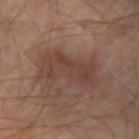Q: Was this lesion biopsied?
A: no biopsy performed (imaged during a skin exam)
Q: Patient demographics?
A: male, aged 68 to 72
Q: What is the imaging modality?
A: 15 mm crop, total-body photography
Q: What did automated image analysis measure?
A: a lesion area of about 16 mm², a shape eccentricity near 0.85, and a shape-asymmetry score of about 0.35 (0 = symmetric); a mean CIELAB color near L≈39 a*≈18 b*≈23, roughly 7 lightness units darker than nearby skin, and a normalized lesion–skin contrast near 6; a classifier nevus-likeness of about 15/100 and a lesion-detection confidence of about 100/100
Q: Where on the body is the lesion?
A: the left forearm
Q: Lesion size?
A: ≈6 mm
Q: Illumination type?
A: cross-polarized